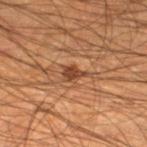Assessment: The lesion was photographed on a routine skin check and not biopsied; there is no pathology result. Background: The lesion-visualizer software estimated a lesion area of about 4 mm², an eccentricity of roughly 0.75, and a symmetry-axis asymmetry near 0.4. The software also gave an average lesion color of about L≈43 a*≈23 b*≈32 (CIELAB), about 11 CIELAB-L* units darker than the surrounding skin, and a lesion-to-skin contrast of about 8 (normalized; higher = more distinct). The software also gave internal color variation of about 2 on a 0–10 scale and radial color variation of about 1. A male patient, roughly 45 years of age. The lesion is located on the left lower leg. This is a cross-polarized tile. The recorded lesion diameter is about 3 mm. Cropped from a total-body skin-imaging series; the visible field is about 15 mm.